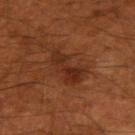{
  "biopsy_status": "not biopsied; imaged during a skin examination",
  "lesion_size": {
    "long_diameter_mm_approx": 5.0
  },
  "image": {
    "source": "total-body photography crop",
    "field_of_view_mm": 15
  },
  "site": "left arm",
  "patient": {
    "sex": "male",
    "age_approx": 50
  },
  "automated_metrics": {
    "cielab_L": 29,
    "cielab_a": 24,
    "cielab_b": 30,
    "vs_skin_darker_L": 8.0,
    "border_irregularity_0_10": 6.0,
    "peripheral_color_asymmetry": 1.0
  }
}